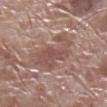Impression: No biopsy was performed on this lesion — it was imaged during a full skin examination and was not determined to be concerning. Image and clinical context: Located on the right lower leg. The lesion's longest dimension is about 5.5 mm. The tile uses white-light illumination. The lesion-visualizer software estimated an area of roughly 14 mm² and two-axis asymmetry of about 0.5. The software also gave a border-irregularity rating of about 7.5/10, a within-lesion color-variation index near 3/10, and radial color variation of about 1. And it measured an automated nevus-likeness rating near 0 out of 100 and a lesion-detection confidence of about 60/100. A male subject, aged around 70. A region of skin cropped from a whole-body photographic capture, roughly 15 mm wide.